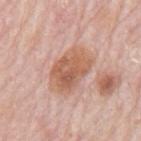follow-up = imaged on a skin check; not biopsied
patient = male, about 80 years old
lesion size = about 6 mm
site = the mid back
lighting = white-light illumination
image = ~15 mm crop, total-body skin-cancer survey